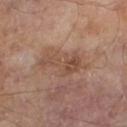notes — total-body-photography surveillance lesion; no biopsy | TBP lesion metrics — a lesion area of about 9 mm², a shape eccentricity near 0.9, and a shape-asymmetry score of about 0.55 (0 = symmetric); about 7 CIELAB-L* units darker than the surrounding skin and a normalized lesion–skin contrast near 6 | acquisition — ~15 mm crop, total-body skin-cancer survey | anatomic site — the left lower leg | lesion diameter — about 5 mm | lighting — cross-polarized illumination | subject — male, approximately 65 years of age.